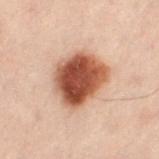Assessment: No biopsy was performed on this lesion — it was imaged during a full skin examination and was not determined to be concerning. Background: A region of skin cropped from a whole-body photographic capture, roughly 15 mm wide. Longest diameter approximately 6 mm. A male subject, aged approximately 50. The lesion is on the left thigh. An algorithmic analysis of the crop reported an average lesion color of about L≈42 a*≈21 b*≈27 (CIELAB), a lesion–skin lightness drop of about 18, and a normalized border contrast of about 13. The software also gave an automated nevus-likeness rating near 100 out of 100 and a lesion-detection confidence of about 100/100.